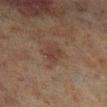Assessment:
Imaged during a routine full-body skin examination; the lesion was not biopsied and no histopathology is available.
Clinical summary:
Captured under cross-polarized illumination. The patient is a male approximately 70 years of age. The recorded lesion diameter is about 3 mm. Automated image analysis of the tile measured an area of roughly 4.5 mm², an eccentricity of roughly 0.75, and a symmetry-axis asymmetry near 0.25. The software also gave an average lesion color of about L≈31 a*≈15 b*≈20 (CIELAB) and roughly 6 lightness units darker than nearby skin. And it measured a classifier nevus-likeness of about 10/100 and lesion-presence confidence of about 100/100. This image is a 15 mm lesion crop taken from a total-body photograph. Located on the right lower leg.